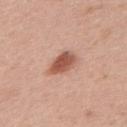The lesion was tiled from a total-body skin photograph and was not biopsied.
A lesion tile, about 15 mm wide, cut from a 3D total-body photograph.
The lesion is located on the back.
A male subject in their mid- to late 60s.